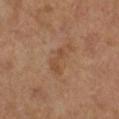Part of a total-body skin-imaging series; this lesion was reviewed on a skin check and was not flagged for biopsy. This image is a 15 mm lesion crop taken from a total-body photograph. The subject is a female roughly 65 years of age. On the right lower leg. Measured at roughly 4 mm in maximum diameter.